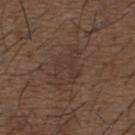A 15 mm close-up extracted from a 3D total-body photography capture. Located on the upper back. Automated image analysis of the tile measured an eccentricity of roughly 0.8 and a symmetry-axis asymmetry near 0.25. The analysis additionally found a classifier nevus-likeness of about 0/100 and a detector confidence of about 95 out of 100 that the crop contains a lesion. Longest diameter approximately 5.5 mm. This is a white-light tile. A male subject, approximately 50 years of age.